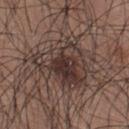<record>
<site>upper back</site>
<image>
  <source>total-body photography crop</source>
  <field_of_view_mm>15</field_of_view_mm>
</image>
<patient>
  <sex>male</sex>
  <age_approx>35</age_approx>
</patient>
</record>